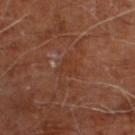The lesion was photographed on a routine skin check and not biopsied; there is no pathology result. Captured under cross-polarized illumination. Automated image analysis of the tile measured an area of roughly 4.5 mm², a shape eccentricity near 0.35, and two-axis asymmetry of about 0.45. It also reported a lesion color around L≈34 a*≈22 b*≈28 in CIELAB, about 4 CIELAB-L* units darker than the surrounding skin, and a normalized border contrast of about 4.5. And it measured an automated nevus-likeness rating near 0 out of 100 and a detector confidence of about 80 out of 100 that the crop contains a lesion. A male patient, aged approximately 60. A 15 mm close-up extracted from a 3D total-body photography capture. The lesion is located on the right leg.